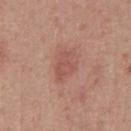Clinical impression: Imaged during a routine full-body skin examination; the lesion was not biopsied and no histopathology is available. Background: Captured under white-light illumination. A 15 mm close-up tile from a total-body photography series done for melanoma screening. The lesion's longest dimension is about 3.5 mm. Automated image analysis of the tile measured a border-irregularity index near 3/10, a color-variation rating of about 2/10, and a peripheral color-asymmetry measure near 0.5. The lesion is on the abdomen. A male subject about 50 years old.